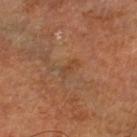Impression:
Recorded during total-body skin imaging; not selected for excision or biopsy.
Acquisition and patient details:
A close-up tile cropped from a whole-body skin photograph, about 15 mm across. The lesion is located on the right leg. The lesion's longest dimension is about 2.5 mm. The subject is a male roughly 60 years of age. The tile uses cross-polarized illumination.